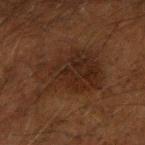image source = total-body-photography crop, ~15 mm field of view; diameter = about 7.5 mm; location = the right upper arm; patient = male, approximately 50 years of age; tile lighting = cross-polarized.